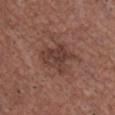Impression:
The lesion was tiled from a total-body skin photograph and was not biopsied.